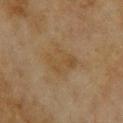| key | value |
|---|---|
| lesion size | ≈4 mm |
| automated lesion analysis | an average lesion color of about L≈44 a*≈13 b*≈32 (CIELAB) and a normalized lesion–skin contrast near 5; a border-irregularity rating of about 3/10 and a color-variation rating of about 3/10; a nevus-likeness score of about 0/100 and lesion-presence confidence of about 100/100 |
| image source | total-body-photography crop, ~15 mm field of view |
| patient | female, about 60 years old |
| location | the back |